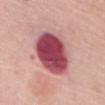Q: Is there a histopathology result?
A: catalogued during a skin exam; not biopsied
Q: Patient demographics?
A: female, aged around 75
Q: Lesion location?
A: the chest
Q: What kind of image is this?
A: total-body-photography crop, ~15 mm field of view